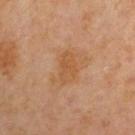notes: no biopsy performed (imaged during a skin exam)
body site: the left upper arm
patient: male, aged approximately 60
image-analysis metrics: an area of roughly 6 mm² and a shape eccentricity near 0.8; an average lesion color of about L≈49 a*≈20 b*≈36 (CIELAB), a lesion–skin lightness drop of about 6, and a normalized border contrast of about 6; a border-irregularity index near 3/10, a within-lesion color-variation index near 2.5/10, and a peripheral color-asymmetry measure near 0.5; a classifier nevus-likeness of about 0/100 and a detector confidence of about 100 out of 100 that the crop contains a lesion
image: 15 mm crop, total-body photography
tile lighting: cross-polarized